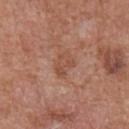workup: no biopsy performed (imaged during a skin exam).